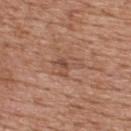Impression: Captured during whole-body skin photography for melanoma surveillance; the lesion was not biopsied. Background: From the upper back. The lesion's longest dimension is about 4 mm. A lesion tile, about 15 mm wide, cut from a 3D total-body photograph. The subject is a male roughly 60 years of age. Captured under white-light illumination.